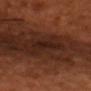notes — total-body-photography surveillance lesion; no biopsy
illumination — cross-polarized illumination
image source — ~15 mm tile from a whole-body skin photo
patient — male, about 50 years old
lesion size — about 3 mm
automated metrics — about 5 CIELAB-L* units darker than the surrounding skin and a lesion-to-skin contrast of about 7 (normalized; higher = more distinct); a color-variation rating of about 1.5/10 and peripheral color asymmetry of about 0.5
site — the head or neck